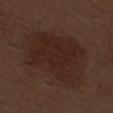biopsy status: total-body-photography surveillance lesion; no biopsy.